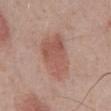The lesion was photographed on a routine skin check and not biopsied; there is no pathology result. The lesion is on the chest. A male subject, aged around 70. A roughly 15 mm field-of-view crop from a total-body skin photograph. Imaged with white-light lighting. Automated tile analysis of the lesion measured a mean CIELAB color near L≈55 a*≈22 b*≈26 and a normalized lesion–skin contrast near 6.5. The analysis additionally found a color-variation rating of about 3.5/10 and peripheral color asymmetry of about 1. The software also gave a classifier nevus-likeness of about 100/100. Measured at roughly 6 mm in maximum diameter.